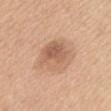The lesion was photographed on a routine skin check and not biopsied; there is no pathology result.
A 15 mm close-up extracted from a 3D total-body photography capture.
From the mid back.
A female subject, in their mid-60s.
The tile uses white-light illumination.